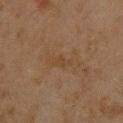workup: catalogued during a skin exam; not biopsied
patient: male, in their mid-40s
image source: total-body-photography crop, ~15 mm field of view
lesion diameter: about 2.5 mm
location: the chest
automated lesion analysis: border irregularity of about 4 on a 0–10 scale
lighting: cross-polarized illumination